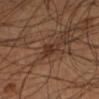The lesion was tiled from a total-body skin photograph and was not biopsied. A male patient, aged around 55. Cropped from a whole-body photographic skin survey; the tile spans about 15 mm. The tile uses cross-polarized illumination. On the left lower leg. Longest diameter approximately 2.5 mm.